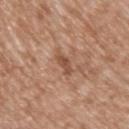Part of a total-body skin-imaging series; this lesion was reviewed on a skin check and was not flagged for biopsy.
This image is a 15 mm lesion crop taken from a total-body photograph.
Measured at roughly 3 mm in maximum diameter.
A male subject, in their mid- to late 60s.
Located on the upper back.